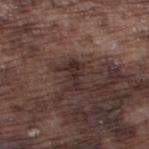Part of a total-body skin-imaging series; this lesion was reviewed on a skin check and was not flagged for biopsy. From the left thigh. The subject is a male aged approximately 75. Cropped from a total-body skin-imaging series; the visible field is about 15 mm.